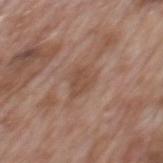biopsy status: catalogued during a skin exam; not biopsied | size: ~3.5 mm (longest diameter) | lighting: white-light | site: the mid back | subject: male, aged around 70 | imaging modality: 15 mm crop, total-body photography.